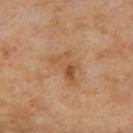Q: Was this lesion biopsied?
A: no biopsy performed (imaged during a skin exam)
Q: What is the imaging modality?
A: total-body-photography crop, ~15 mm field of view
Q: What lighting was used for the tile?
A: cross-polarized
Q: Who is the patient?
A: male, aged 68–72
Q: What is the anatomic site?
A: the mid back
Q: What is the lesion's diameter?
A: about 4.5 mm